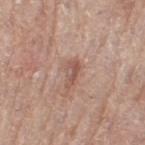Q: Was this lesion biopsied?
A: imaged on a skin check; not biopsied
Q: What lighting was used for the tile?
A: white-light illumination
Q: Who is the patient?
A: female, roughly 65 years of age
Q: How was this image acquired?
A: ~15 mm tile from a whole-body skin photo
Q: Lesion size?
A: ≈3 mm
Q: Where on the body is the lesion?
A: the leg